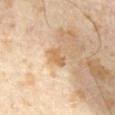notes: catalogued during a skin exam; not biopsied | subject: male, in their mid- to late 60s | lesion size: ~2.5 mm (longest diameter) | imaging modality: 15 mm crop, total-body photography | lighting: cross-polarized illumination | automated metrics: a footprint of about 3 mm² and a symmetry-axis asymmetry near 0.35; border irregularity of about 3.5 on a 0–10 scale and a peripheral color-asymmetry measure near 0.